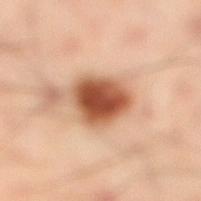Imaged during a routine full-body skin examination; the lesion was not biopsied and no histopathology is available.
A male patient aged around 40.
A roughly 15 mm field-of-view crop from a total-body skin photograph.
The lesion is on the right lower leg.